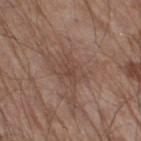Captured under white-light illumination. The patient is a male aged 78–82. Longest diameter approximately 3.5 mm. This image is a 15 mm lesion crop taken from a total-body photograph. Located on the right thigh.